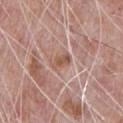The lesion was tiled from a total-body skin photograph and was not biopsied.
A male subject, aged approximately 70.
An algorithmic analysis of the crop reported a border-irregularity index near 3/10. And it measured a detector confidence of about 85 out of 100 that the crop contains a lesion.
From the chest.
Captured under white-light illumination.
Longest diameter approximately 3 mm.
A 15 mm close-up extracted from a 3D total-body photography capture.